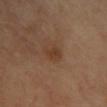biopsy status: total-body-photography surveillance lesion; no biopsy
subject: female, aged around 40
body site: the front of the torso
automated lesion analysis: an area of roughly 4 mm², an eccentricity of roughly 0.7, and a shape-asymmetry score of about 0.2 (0 = symmetric)
acquisition: ~15 mm crop, total-body skin-cancer survey